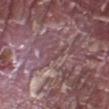Assessment:
Recorded during total-body skin imaging; not selected for excision or biopsy.
Acquisition and patient details:
A 15 mm close-up tile from a total-body photography series done for melanoma screening. The tile uses white-light illumination. A male subject, aged approximately 40. Approximately 2.5 mm at its widest. On the right upper arm.